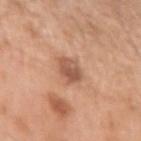Case summary:
* follow-up — no biopsy performed (imaged during a skin exam)
* lighting — white-light illumination
* image-analysis metrics — an area of roughly 6 mm² and a shape-asymmetry score of about 0.2 (0 = symmetric); roughly 12 lightness units darker than nearby skin and a lesion-to-skin contrast of about 8 (normalized; higher = more distinct); a border-irregularity index near 2/10 and peripheral color asymmetry of about 1.5; a nevus-likeness score of about 25/100 and a detector confidence of about 100 out of 100 that the crop contains a lesion
* image — ~15 mm tile from a whole-body skin photo
* site — the left upper arm
* subject — male, approximately 55 years of age